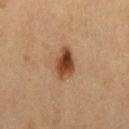Assessment: The lesion was tiled from a total-body skin photograph and was not biopsied. Background: The patient is a female about 40 years old. About 4 mm across. The lesion-visualizer software estimated a border-irregularity index near 2/10 and a color-variation rating of about 6.5/10. The software also gave an automated nevus-likeness rating near 100 out of 100 and a detector confidence of about 100 out of 100 that the crop contains a lesion. On the right thigh. Imaged with cross-polarized lighting. Cropped from a whole-body photographic skin survey; the tile spans about 15 mm.